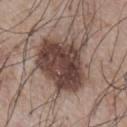<tbp_lesion>
<automated_metrics>
  <area_mm2_approx>33.0</area_mm2_approx>
  <eccentricity>0.55</eccentricity>
  <shape_asymmetry>0.25</shape_asymmetry>
  <cielab_L>43</cielab_L>
  <cielab_a>16</cielab_a>
  <cielab_b>21</cielab_b>
  <vs_skin_darker_L>15.0</vs_skin_darker_L>
  <border_irregularity_0_10>4.0</border_irregularity_0_10>
  <color_variation_0_10>6.0</color_variation_0_10>
  <peripheral_color_asymmetry>2.0</peripheral_color_asymmetry>
</automated_metrics>
<site>chest</site>
<patient>
  <sex>male</sex>
  <age_approx>75</age_approx>
</patient>
<image>
  <source>total-body photography crop</source>
  <field_of_view_mm>15</field_of_view_mm>
</image>
<lesion_size>
  <long_diameter_mm_approx>7.5</long_diameter_mm_approx>
</lesion_size>
</tbp_lesion>